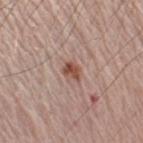Findings:
* biopsy status: imaged on a skin check; not biopsied
* site: the right upper arm
* patient: male, aged 63 to 67
* image source: total-body-photography crop, ~15 mm field of view